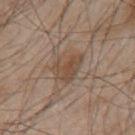Case summary:
* notes: catalogued during a skin exam; not biopsied